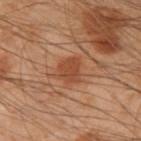| field | value |
|---|---|
| workup | no biopsy performed (imaged during a skin exam) |
| imaging modality | ~15 mm tile from a whole-body skin photo |
| lesion size | ~3.5 mm (longest diameter) |
| anatomic site | the left forearm |
| subject | male, aged around 50 |
| automated lesion analysis | an outline eccentricity of about 0.75 (0 = round, 1 = elongated) and a shape-asymmetry score of about 0.2 (0 = symmetric); a border-irregularity index near 2.5/10 and peripheral color asymmetry of about 0.5; an automated nevus-likeness rating near 70 out of 100 |